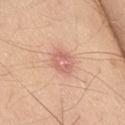Notes:
- patient — male, approximately 65 years of age
- image-analysis metrics — a mean CIELAB color near L≈63 a*≈26 b*≈28 and a lesion-to-skin contrast of about 6 (normalized; higher = more distinct); a border-irregularity index near 2/10 and peripheral color asymmetry of about 1; lesion-presence confidence of about 100/100
- location — the right thigh
- imaging modality — 15 mm crop, total-body photography
- illumination — white-light